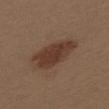Notes:
• biopsy status: no biopsy performed (imaged during a skin exam)
• subject: female, approximately 40 years of age
• body site: the upper back
• image source: ~15 mm crop, total-body skin-cancer survey